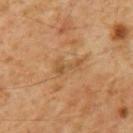This lesion was catalogued during total-body skin photography and was not selected for biopsy.
Automated image analysis of the tile measured a mean CIELAB color near L≈48 a*≈18 b*≈36, a lesion–skin lightness drop of about 7, and a lesion-to-skin contrast of about 5.5 (normalized; higher = more distinct).
The lesion is on the mid back.
A male subject, aged 58–62.
Cropped from a total-body skin-imaging series; the visible field is about 15 mm.
Captured under cross-polarized illumination.